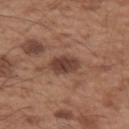notes — no biopsy performed (imaged during a skin exam) | site — the left upper arm | image-analysis metrics — a nevus-likeness score of about 55/100 and a detector confidence of about 100 out of 100 that the crop contains a lesion | image source — ~15 mm crop, total-body skin-cancer survey | illumination — white-light illumination | subject — male, in their mid- to late 50s.